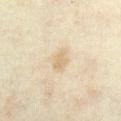Recorded during total-body skin imaging; not selected for excision or biopsy.
Located on the abdomen.
The lesion's longest dimension is about 2.5 mm.
An algorithmic analysis of the crop reported border irregularity of about 2 on a 0–10 scale, a within-lesion color-variation index near 1.5/10, and peripheral color asymmetry of about 0.5. The analysis additionally found an automated nevus-likeness rating near 0 out of 100 and lesion-presence confidence of about 95/100.
A lesion tile, about 15 mm wide, cut from a 3D total-body photograph.
The patient is a female in their mid-30s.
Captured under cross-polarized illumination.